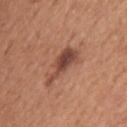Part of a total-body skin-imaging series; this lesion was reviewed on a skin check and was not flagged for biopsy. A male patient aged approximately 65. This is a white-light tile. Longest diameter approximately 5.5 mm. The total-body-photography lesion software estimated a mean CIELAB color near L≈45 a*≈23 b*≈28, a lesion–skin lightness drop of about 13, and a lesion-to-skin contrast of about 9.5 (normalized; higher = more distinct). And it measured a nevus-likeness score of about 60/100 and lesion-presence confidence of about 100/100. A lesion tile, about 15 mm wide, cut from a 3D total-body photograph. On the upper back.